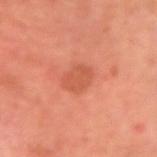Background:
The patient is a male aged around 65. The lesion is located on the right upper arm. A region of skin cropped from a whole-body photographic capture, roughly 15 mm wide.